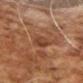A lesion tile, about 15 mm wide, cut from a 3D total-body photograph. The lesion is on the right upper arm. The patient is a male aged 68 to 72.